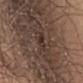Impression:
No biopsy was performed on this lesion — it was imaged during a full skin examination and was not determined to be concerning.
Background:
This is a white-light tile. Automated image analysis of the tile measured a footprint of about 3.5 mm², an eccentricity of roughly 0.8, and a shape-asymmetry score of about 0.35 (0 = symmetric). It also reported internal color variation of about 3.5 on a 0–10 scale. A region of skin cropped from a whole-body photographic capture, roughly 15 mm wide. A male patient aged around 45. The lesion is located on the chest.